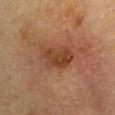No biopsy was performed on this lesion — it was imaged during a full skin examination and was not determined to be concerning. This image is a 15 mm lesion crop taken from a total-body photograph. The subject is a male roughly 65 years of age. Located on the left upper arm. About 4 mm across. Automated tile analysis of the lesion measured a lesion area of about 10 mm², an outline eccentricity of about 0.65 (0 = round, 1 = elongated), and two-axis asymmetry of about 0.25. It also reported a lesion–skin lightness drop of about 7 and a normalized border contrast of about 7.5.